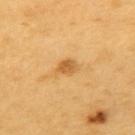{
  "biopsy_status": "not biopsied; imaged during a skin examination",
  "patient": {
    "sex": "male",
    "age_approx": 60
  },
  "image": {
    "source": "total-body photography crop",
    "field_of_view_mm": 15
  },
  "site": "left upper arm"
}